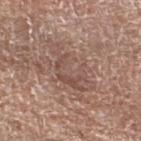  biopsy_status: not biopsied; imaged during a skin examination
  image:
    source: total-body photography crop
    field_of_view_mm: 15
  site: left lower leg
  patient:
    sex: female
    age_approx: 75